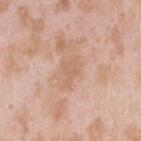{
  "biopsy_status": "not biopsied; imaged during a skin examination",
  "image": {
    "source": "total-body photography crop",
    "field_of_view_mm": 15
  },
  "automated_metrics": {
    "cielab_L": 63,
    "cielab_a": 19,
    "cielab_b": 31,
    "vs_skin_darker_L": 7.0,
    "vs_skin_contrast_norm": 5.0,
    "border_irregularity_0_10": 4.5,
    "nevus_likeness_0_100": 0,
    "lesion_detection_confidence_0_100": 100
  },
  "patient": {
    "sex": "female",
    "age_approx": 25
  },
  "lesion_size": {
    "long_diameter_mm_approx": 3.5
  },
  "lighting": "white-light",
  "site": "right upper arm"
}